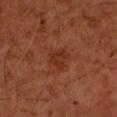Impression:
Imaged during a routine full-body skin examination; the lesion was not biopsied and no histopathology is available.
Image and clinical context:
A 15 mm close-up extracted from a 3D total-body photography capture. An algorithmic analysis of the crop reported a footprint of about 6 mm², an eccentricity of roughly 0.5, and a symmetry-axis asymmetry near 0.4. It also reported a lesion color around L≈30 a*≈25 b*≈30 in CIELAB, about 6 CIELAB-L* units darker than the surrounding skin, and a lesion-to-skin contrast of about 6 (normalized; higher = more distinct). The software also gave a nevus-likeness score of about 0/100 and lesion-presence confidence of about 100/100. Imaged with cross-polarized lighting. The lesion is located on the left forearm. A male patient about 60 years old. The recorded lesion diameter is about 3 mm.